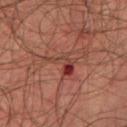Imaged during a routine full-body skin examination; the lesion was not biopsied and no histopathology is available. The lesion's longest dimension is about 4.5 mm. Cropped from a total-body skin-imaging series; the visible field is about 15 mm. The tile uses cross-polarized illumination. The subject is a male aged 63 to 67. On the chest.